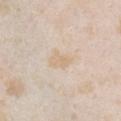Impression: Part of a total-body skin-imaging series; this lesion was reviewed on a skin check and was not flagged for biopsy. Image and clinical context: A 15 mm close-up extracted from a 3D total-body photography capture. Located on the chest. A female subject aged approximately 25.